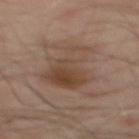Case summary:
– biopsy status · total-body-photography surveillance lesion; no biopsy